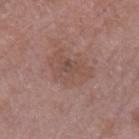Assessment: The lesion was tiled from a total-body skin photograph and was not biopsied. Background: Longest diameter approximately 5.5 mm. This image is a 15 mm lesion crop taken from a total-body photograph. The lesion-visualizer software estimated a shape eccentricity near 0.8 and a shape-asymmetry score of about 0.3 (0 = symmetric). The software also gave a lesion color around L≈49 a*≈19 b*≈24 in CIELAB and a lesion-to-skin contrast of about 5 (normalized; higher = more distinct). It also reported border irregularity of about 3.5 on a 0–10 scale. The analysis additionally found an automated nevus-likeness rating near 0 out of 100. The subject is a male about 75 years old. From the left forearm.